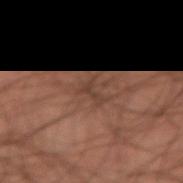This lesion was catalogued during total-body skin photography and was not selected for biopsy.
This image is a 15 mm lesion crop taken from a total-body photograph.
The patient is a male aged 43 to 47.
About 2.5 mm across.
From the abdomen.
Imaged with cross-polarized lighting.